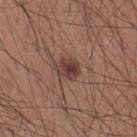Clinical impression: This lesion was catalogued during total-body skin photography and was not selected for biopsy. Clinical summary: On the mid back. The total-body-photography lesion software estimated a shape-asymmetry score of about 0.15 (0 = symmetric). The analysis additionally found a nevus-likeness score of about 95/100. A lesion tile, about 15 mm wide, cut from a 3D total-body photograph. A male subject aged 33 to 37. Approximately 3 mm at its widest. Captured under white-light illumination.